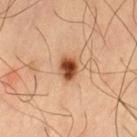notes = total-body-photography surveillance lesion; no biopsy | image = 15 mm crop, total-body photography | size = about 3 mm | subject = male, aged 58 to 62 | illumination = cross-polarized illumination | anatomic site = the leg.